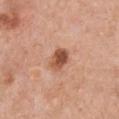biopsy status: total-body-photography surveillance lesion; no biopsy
acquisition: ~15 mm tile from a whole-body skin photo
lesion diameter: ≈3 mm
subject: female, in their 60s
anatomic site: the chest